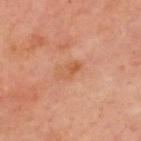Notes:
• follow-up: no biopsy performed (imaged during a skin exam)
• image source: total-body-photography crop, ~15 mm field of view
• location: the upper back
• patient: male, aged 48–52